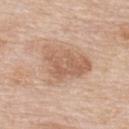Findings:
– site — the back
– patient — male, approximately 85 years of age or older
– image source — total-body-photography crop, ~15 mm field of view